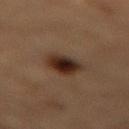Assessment:
This lesion was catalogued during total-body skin photography and was not selected for biopsy.
Background:
The subject is a male approximately 85 years of age or older. The lesion is on the mid back. A 15 mm close-up tile from a total-body photography series done for melanoma screening.